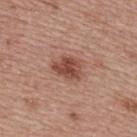The lesion-visualizer software estimated border irregularity of about 3 on a 0–10 scale, internal color variation of about 3 on a 0–10 scale, and radial color variation of about 1. The lesion is on the upper back. The subject is a female about 65 years old. A 15 mm close-up extracted from a 3D total-body photography capture. This is a white-light tile.